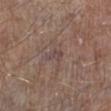<lesion>
<image>
  <source>total-body photography crop</source>
  <field_of_view_mm>15</field_of_view_mm>
</image>
<patient>
  <sex>male</sex>
  <age_approx>55</age_approx>
</patient>
<site>left lower leg</site>
</lesion>